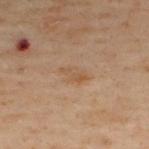Findings:
• illumination — cross-polarized
• patient — male, in their 50s
• size — ≈3 mm
• site — the upper back
• acquisition — ~15 mm tile from a whole-body skin photo